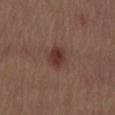The patient is a male aged around 70. The lesion is located on the back. The total-body-photography lesion software estimated a detector confidence of about 100 out of 100 that the crop contains a lesion. Cropped from a whole-body photographic skin survey; the tile spans about 15 mm.